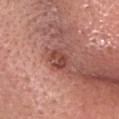Captured during whole-body skin photography for melanoma surveillance; the lesion was not biopsied. This image is a 15 mm lesion crop taken from a total-body photograph. The lesion is located on the head or neck. A female patient, approximately 65 years of age.